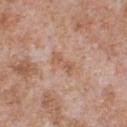Captured during whole-body skin photography for melanoma surveillance; the lesion was not biopsied.
From the front of the torso.
A region of skin cropped from a whole-body photographic capture, roughly 15 mm wide.
The lesion's longest dimension is about 3 mm.
A male patient roughly 65 years of age.